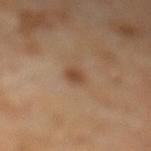follow-up=total-body-photography surveillance lesion; no biopsy
image source=15 mm crop, total-body photography
patient=male, in their mid- to late 60s
anatomic site=the leg